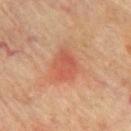| key | value |
|---|---|
| workup | imaged on a skin check; not biopsied |
| image | total-body-photography crop, ~15 mm field of view |
| subject | male, roughly 75 years of age |
| size | about 4 mm |
| anatomic site | the chest |
| illumination | cross-polarized illumination |